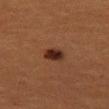Assessment: Part of a total-body skin-imaging series; this lesion was reviewed on a skin check and was not flagged for biopsy. Acquisition and patient details: A close-up tile cropped from a whole-body skin photograph, about 15 mm across. The tile uses cross-polarized illumination. The lesion-visualizer software estimated a footprint of about 4 mm², an eccentricity of roughly 0.7, and a shape-asymmetry score of about 0.2 (0 = symmetric). It also reported border irregularity of about 2 on a 0–10 scale, a color-variation rating of about 3.5/10, and a peripheral color-asymmetry measure near 1. The recorded lesion diameter is about 2.5 mm. A female subject about 55 years old. The lesion is on the left thigh.